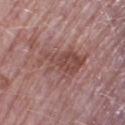patient: female, roughly 70 years of age | imaging modality: 15 mm crop, total-body photography | automated metrics: a footprint of about 15 mm², an eccentricity of roughly 0.85, and a shape-asymmetry score of about 0.4 (0 = symmetric); an average lesion color of about L≈47 a*≈22 b*≈23 (CIELAB), about 9 CIELAB-L* units darker than the surrounding skin, and a normalized lesion–skin contrast near 7; an automated nevus-likeness rating near 0 out of 100 and a detector confidence of about 95 out of 100 that the crop contains a lesion | location: the right thigh | tile lighting: white-light | lesion diameter: ~7 mm (longest diameter).